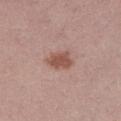Background:
This is a white-light tile. The total-body-photography lesion software estimated an automated nevus-likeness rating near 90 out of 100 and lesion-presence confidence of about 100/100. The lesion is on the leg. The subject is a female aged around 40. Cropped from a whole-body photographic skin survey; the tile spans about 15 mm.